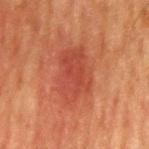{"biopsy_status": "not biopsied; imaged during a skin examination", "patient": {"sex": "male", "age_approx": 60}, "automated_metrics": {"area_mm2_approx": 17.0, "eccentricity": 0.8, "shape_asymmetry": 0.25, "vs_skin_darker_L": 7.0, "vs_skin_contrast_norm": 6.0, "nevus_likeness_0_100": 90}, "image": {"source": "total-body photography crop", "field_of_view_mm": 15}, "lesion_size": {"long_diameter_mm_approx": 6.0}, "site": "left upper arm", "lighting": "cross-polarized"}